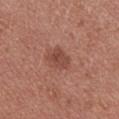Clinical impression: The lesion was tiled from a total-body skin photograph and was not biopsied. Clinical summary: A 15 mm close-up tile from a total-body photography series done for melanoma screening. Approximately 3 mm at its widest. This is a white-light tile. From the back. A male patient aged 23 to 27.